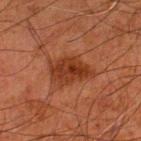Part of a total-body skin-imaging series; this lesion was reviewed on a skin check and was not flagged for biopsy. The lesion is on the left lower leg. The subject is a male in their 80s. A region of skin cropped from a whole-body photographic capture, roughly 15 mm wide. Longest diameter approximately 4.5 mm. Imaged with cross-polarized lighting. Automated image analysis of the tile measured a nevus-likeness score of about 85/100 and a detector confidence of about 100 out of 100 that the crop contains a lesion.